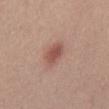{"biopsy_status": "not biopsied; imaged during a skin examination", "site": "back", "lighting": "white-light", "automated_metrics": {"color_variation_0_10": 2.5, "peripheral_color_asymmetry": 1.0, "nevus_likeness_0_100": 90, "lesion_detection_confidence_0_100": 100}, "patient": {"sex": "male", "age_approx": 55}, "image": {"source": "total-body photography crop", "field_of_view_mm": 15}}